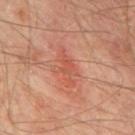• notes: imaged on a skin check; not biopsied
• illumination: cross-polarized illumination
• imaging modality: ~15 mm tile from a whole-body skin photo
• diameter: ≈4 mm
• anatomic site: the mid back
• automated metrics: an average lesion color of about L≈53 a*≈30 b*≈32 (CIELAB), roughly 8 lightness units darker than nearby skin, and a normalized lesion–skin contrast near 5.5; a border-irregularity index near 5.5/10 and a peripheral color-asymmetry measure near 1.5
• subject: male, aged around 65